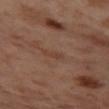notes: no biopsy performed (imaged during a skin exam)
anatomic site: the left thigh
subject: female, in their mid-50s
imaging modality: ~15 mm tile from a whole-body skin photo
tile lighting: cross-polarized illumination
automated metrics: a lesion area of about 3 mm² and a shape eccentricity near 0.9; an automated nevus-likeness rating near 0 out of 100 and a lesion-detection confidence of about 100/100
lesion diameter: ≈3 mm